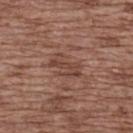Impression: This lesion was catalogued during total-body skin photography and was not selected for biopsy. Background: On the upper back. A female subject in their mid- to late 70s. The lesion's longest dimension is about 4 mm. A 15 mm crop from a total-body photograph taken for skin-cancer surveillance. Imaged with white-light lighting. The total-body-photography lesion software estimated an area of roughly 5.5 mm² and two-axis asymmetry of about 0.5. The analysis additionally found an average lesion color of about L≈43 a*≈21 b*≈26 (CIELAB), roughly 8 lightness units darker than nearby skin, and a normalized border contrast of about 6. It also reported a color-variation rating of about 1.5/10 and a peripheral color-asymmetry measure near 0.5. The analysis additionally found a classifier nevus-likeness of about 0/100 and lesion-presence confidence of about 85/100.